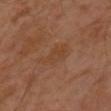workup=catalogued during a skin exam; not biopsied.